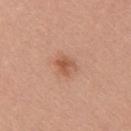Imaged during a routine full-body skin examination; the lesion was not biopsied and no histopathology is available.
A female subject, roughly 35 years of age.
From the arm.
The lesion's longest dimension is about 3 mm.
Captured under white-light illumination.
The lesion-visualizer software estimated a lesion area of about 4.5 mm² and a symmetry-axis asymmetry near 0.3. The software also gave a lesion color around L≈56 a*≈24 b*≈33 in CIELAB, roughly 9 lightness units darker than nearby skin, and a lesion-to-skin contrast of about 6.5 (normalized; higher = more distinct). And it measured border irregularity of about 3 on a 0–10 scale, a color-variation rating of about 3.5/10, and a peripheral color-asymmetry measure near 1. And it measured a nevus-likeness score of about 70/100 and a lesion-detection confidence of about 100/100.
A lesion tile, about 15 mm wide, cut from a 3D total-body photograph.